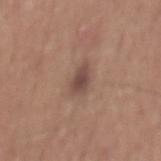{
  "patient": {
    "sex": "male",
    "age_approx": 65
  },
  "automated_metrics": {
    "area_mm2_approx": 4.5,
    "eccentricity": 0.85,
    "shape_asymmetry": 0.3,
    "border_irregularity_0_10": 3.0,
    "color_variation_0_10": 2.0,
    "peripheral_color_asymmetry": 0.5,
    "nevus_likeness_0_100": 85,
    "lesion_detection_confidence_0_100": 100
  },
  "image": {
    "source": "total-body photography crop",
    "field_of_view_mm": 15
  },
  "lighting": "white-light",
  "site": "mid back"
}